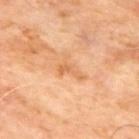biopsy status = catalogued during a skin exam; not biopsied | site = the upper back | automated lesion analysis = a mean CIELAB color near L≈64 a*≈24 b*≈40, a lesion–skin lightness drop of about 7, and a normalized lesion–skin contrast near 5.5 | lighting = cross-polarized | subject = male, aged 63–67 | image source = ~15 mm crop, total-body skin-cancer survey | size = ~3.5 mm (longest diameter).